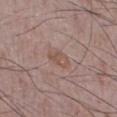Findings:
* biopsy status — catalogued during a skin exam; not biopsied
* image — total-body-photography crop, ~15 mm field of view
* site — the leg
* patient — male, in their mid- to late 70s
* diameter — ≈3.5 mm
* TBP lesion metrics — border irregularity of about 2 on a 0–10 scale, internal color variation of about 3 on a 0–10 scale, and a peripheral color-asymmetry measure near 1; a detector confidence of about 100 out of 100 that the crop contains a lesion
* lighting — white-light illumination